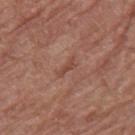This lesion was catalogued during total-body skin photography and was not selected for biopsy. The patient is a female aged approximately 80. Cropped from a whole-body photographic skin survey; the tile spans about 15 mm. The lesion is on the right thigh. Automated image analysis of the tile measured a symmetry-axis asymmetry near 0.45. The software also gave about 7 CIELAB-L* units darker than the surrounding skin and a normalized lesion–skin contrast near 5.5. The analysis additionally found border irregularity of about 5 on a 0–10 scale, a within-lesion color-variation index near 0/10, and peripheral color asymmetry of about 0. The software also gave a nevus-likeness score of about 0/100 and a lesion-detection confidence of about 100/100.